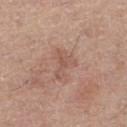Case summary:
– follow-up — total-body-photography surveillance lesion; no biopsy
– patient — female, roughly 50 years of age
– lesion diameter — ~4 mm (longest diameter)
– tile lighting — white-light illumination
– anatomic site — the left thigh
– imaging modality — total-body-photography crop, ~15 mm field of view